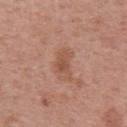Imaged during a routine full-body skin examination; the lesion was not biopsied and no histopathology is available. Located on the left upper arm. A lesion tile, about 15 mm wide, cut from a 3D total-body photograph. The subject is a female aged approximately 40.